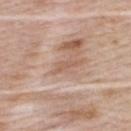Notes:
– workup · total-body-photography surveillance lesion; no biopsy
– anatomic site · the upper back
– tile lighting · white-light
– subject · female, aged 43 to 47
– lesion size · ≈3 mm
– acquisition · 15 mm crop, total-body photography